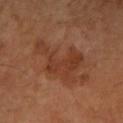follow-up — total-body-photography surveillance lesion; no biopsy | imaging modality — total-body-photography crop, ~15 mm field of view | lesion diameter — about 6.5 mm | lighting — cross-polarized illumination | body site — the arm | patient — female, aged approximately 70.